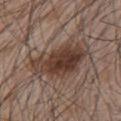Imaged during a routine full-body skin examination; the lesion was not biopsied and no histopathology is available.
On the chest.
A 15 mm close-up tile from a total-body photography series done for melanoma screening.
Imaged with white-light lighting.
A male subject, aged approximately 65.
The lesion-visualizer software estimated a lesion area of about 24 mm² and an outline eccentricity of about 0.85 (0 = round, 1 = elongated). It also reported a border-irregularity rating of about 3.5/10, a color-variation rating of about 6.5/10, and radial color variation of about 2.5. It also reported a classifier nevus-likeness of about 95/100 and lesion-presence confidence of about 100/100.